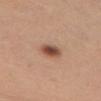Impression:
This lesion was catalogued during total-body skin photography and was not selected for biopsy.
Background:
Automated image analysis of the tile measured a lesion color around L≈50 a*≈21 b*≈30 in CIELAB, roughly 15 lightness units darker than nearby skin, and a normalized border contrast of about 10. Cropped from a total-body skin-imaging series; the visible field is about 15 mm. About 3 mm across. The lesion is on the chest. A female subject aged 53 to 57.